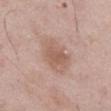  biopsy_status: not biopsied; imaged during a skin examination
  site: abdomen
  lighting: white-light
  image:
    source: total-body photography crop
    field_of_view_mm: 15
  patient:
    sex: male
    age_approx: 50
  lesion_size:
    long_diameter_mm_approx: 4.5